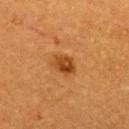An algorithmic analysis of the crop reported an area of roughly 5.5 mm² and an eccentricity of roughly 0.7. It also reported a mean CIELAB color near L≈39 a*≈24 b*≈38, about 10 CIELAB-L* units darker than the surrounding skin, and a normalized border contrast of about 8.5.
The patient is a female approximately 55 years of age.
About 3 mm across.
Imaged with cross-polarized lighting.
A 15 mm crop from a total-body photograph taken for skin-cancer surveillance.
Located on the upper back.